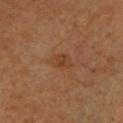Recorded during total-body skin imaging; not selected for excision or biopsy.
Approximately 2.5 mm at its widest.
A region of skin cropped from a whole-body photographic capture, roughly 15 mm wide.
Automated tile analysis of the lesion measured an area of roughly 4 mm² and a symmetry-axis asymmetry near 0.2. It also reported an average lesion color of about L≈38 a*≈20 b*≈32 (CIELAB), roughly 5 lightness units darker than nearby skin, and a lesion-to-skin contrast of about 5.5 (normalized; higher = more distinct). It also reported an automated nevus-likeness rating near 0 out of 100 and lesion-presence confidence of about 100/100.
Imaged with cross-polarized lighting.
The lesion is located on the arm.
The patient is a female aged 58 to 62.